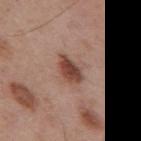* follow-up: catalogued during a skin exam; not biopsied
* lesion size: ≈3.5 mm
* patient: male, aged approximately 55
* imaging modality: ~15 mm tile from a whole-body skin photo
* automated lesion analysis: a lesion color around L≈45 a*≈22 b*≈26 in CIELAB and a normalized border contrast of about 10; a lesion-detection confidence of about 100/100
* body site: the back